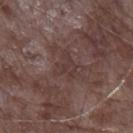Q: Was a biopsy performed?
A: catalogued during a skin exam; not biopsied
Q: What kind of image is this?
A: 15 mm crop, total-body photography
Q: Who is the patient?
A: male, about 65 years old
Q: What is the anatomic site?
A: the arm
Q: Lesion size?
A: about 3 mm